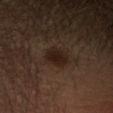Recorded during total-body skin imaging; not selected for excision or biopsy.
The subject is a female approximately 50 years of age.
A lesion tile, about 15 mm wide, cut from a 3D total-body photograph.
Automated image analysis of the tile measured an area of roughly 6.5 mm² and a shape-asymmetry score of about 0.2 (0 = symmetric). And it measured lesion-presence confidence of about 100/100.
The tile uses cross-polarized illumination.